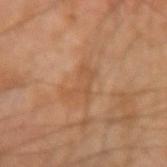Background: The patient is a male in their mid-40s. The recorded lesion diameter is about 3.5 mm. A roughly 15 mm field-of-view crop from a total-body skin photograph. Captured under cross-polarized illumination. From the left forearm.